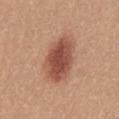{"biopsy_status": "not biopsied; imaged during a skin examination", "lighting": "white-light", "lesion_size": {"long_diameter_mm_approx": 6.5}, "image": {"source": "total-body photography crop", "field_of_view_mm": 15}, "site": "mid back", "patient": {"sex": "female", "age_approx": 30}}